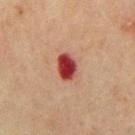Q: Was this lesion biopsied?
A: total-body-photography surveillance lesion; no biopsy
Q: What is the imaging modality?
A: ~15 mm tile from a whole-body skin photo
Q: Lesion size?
A: ≈3 mm
Q: What are the patient's age and sex?
A: male, about 70 years old
Q: What did automated image analysis measure?
A: a border-irregularity index near 1.5/10 and a color-variation rating of about 3.5/10; a classifier nevus-likeness of about 0/100
Q: What lighting was used for the tile?
A: cross-polarized
Q: Lesion location?
A: the mid back